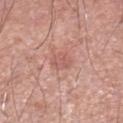  biopsy_status: not biopsied; imaged during a skin examination
  site: left lower leg
  automated_metrics:
    border_irregularity_0_10: 2.0
    color_variation_0_10: 2.0
    peripheral_color_asymmetry: 0.5
    nevus_likeness_0_100: 0
    lesion_detection_confidence_0_100: 100
  lesion_size:
    long_diameter_mm_approx: 2.5
  image:
    source: total-body photography crop
    field_of_view_mm: 15
  patient:
    sex: male
    age_approx: 55
  lighting: white-light